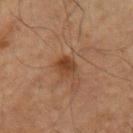Clinical impression:
This lesion was catalogued during total-body skin photography and was not selected for biopsy.
Image and clinical context:
A male patient, aged around 65. A lesion tile, about 15 mm wide, cut from a 3D total-body photograph. The lesion is on the left upper arm. Longest diameter approximately 2.5 mm. Automated image analysis of the tile measured a lesion color around L≈33 a*≈18 b*≈28 in CIELAB, about 8 CIELAB-L* units darker than the surrounding skin, and a normalized border contrast of about 8. The analysis additionally found a lesion-detection confidence of about 100/100.